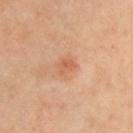Captured during whole-body skin photography for melanoma surveillance; the lesion was not biopsied.
Imaged with cross-polarized lighting.
A male subject in their mid- to late 40s.
The total-body-photography lesion software estimated roughly 8 lightness units darker than nearby skin and a normalized lesion–skin contrast near 5.5. The software also gave border irregularity of about 3 on a 0–10 scale, a within-lesion color-variation index near 2/10, and radial color variation of about 0.5. The analysis additionally found a nevus-likeness score of about 35/100.
Measured at roughly 2.5 mm in maximum diameter.
A 15 mm close-up extracted from a 3D total-body photography capture.
Located on the chest.